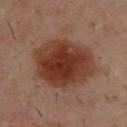{"biopsy_status": "not biopsied; imaged during a skin examination", "automated_metrics": {"area_mm2_approx": 34.0, "eccentricity": 0.55, "shape_asymmetry": 0.15, "color_variation_0_10": 5.0, "peripheral_color_asymmetry": 1.5}, "image": {"source": "total-body photography crop", "field_of_view_mm": 15}, "site": "upper back", "patient": {"sex": "male", "age_approx": 40}}